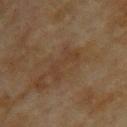<lesion>
  <biopsy_status>not biopsied; imaged during a skin examination</biopsy_status>
  <lesion_size>
    <long_diameter_mm_approx>4.5</long_diameter_mm_approx>
  </lesion_size>
  <lighting>cross-polarized</lighting>
  <site>upper back</site>
  <automated_metrics>
    <area_mm2_approx>6.5</area_mm2_approx>
    <eccentricity>0.9</eccentricity>
    <shape_asymmetry>0.4</shape_asymmetry>
  </automated_metrics>
  <patient>
    <sex>female</sex>
    <age_approx>60</age_approx>
  </patient>
  <image>
    <source>total-body photography crop</source>
    <field_of_view_mm>15</field_of_view_mm>
  </image>
</lesion>